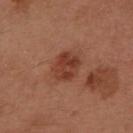Notes:
- diameter: ~3.5 mm (longest diameter)
- imaging modality: 15 mm crop, total-body photography
- body site: the left forearm
- subject: female, approximately 60 years of age
- TBP lesion metrics: a footprint of about 8.5 mm², an outline eccentricity of about 0.6 (0 = round, 1 = elongated), and a shape-asymmetry score of about 0.15 (0 = symmetric); a lesion color around L≈40 a*≈26 b*≈31 in CIELAB and a lesion–skin lightness drop of about 9; an automated nevus-likeness rating near 55 out of 100 and a detector confidence of about 100 out of 100 that the crop contains a lesion